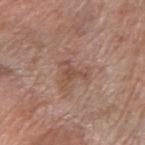{"biopsy_status": "not biopsied; imaged during a skin examination", "image": {"source": "total-body photography crop", "field_of_view_mm": 15}, "lighting": "white-light", "site": "right forearm", "patient": {"sex": "female", "age_approx": 70}, "automated_metrics": {"area_mm2_approx": 3.5, "eccentricity": 0.7, "shape_asymmetry": 0.75, "cielab_L": 49, "cielab_a": 20, "cielab_b": 27, "vs_skin_contrast_norm": 6.0, "color_variation_0_10": 1.0, "peripheral_color_asymmetry": 0.0}, "lesion_size": {"long_diameter_mm_approx": 3.5}}